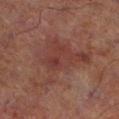Imaged during a routine full-body skin examination; the lesion was not biopsied and no histopathology is available. A male subject in their 70s. Located on the left lower leg. A 15 mm close-up tile from a total-body photography series done for melanoma screening.